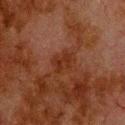Recorded during total-body skin imaging; not selected for excision or biopsy. A male patient aged 78–82. The tile uses cross-polarized illumination. The lesion is located on the upper back. A 15 mm crop from a total-body photograph taken for skin-cancer surveillance. Automated tile analysis of the lesion measured a lesion area of about 5 mm², a shape eccentricity near 0.55, and a symmetry-axis asymmetry near 0.3. And it measured a border-irregularity index near 4/10 and a peripheral color-asymmetry measure near 1.